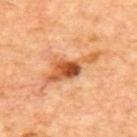No biopsy was performed on this lesion — it was imaged during a full skin examination and was not determined to be concerning. A patient aged approximately 65. This image is a 15 mm lesion crop taken from a total-body photograph. The lesion is on the mid back.